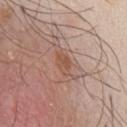The lesion-visualizer software estimated a shape eccentricity near 0.85 and a symmetry-axis asymmetry near 0.3. It also reported border irregularity of about 3 on a 0–10 scale, internal color variation of about 2 on a 0–10 scale, and peripheral color asymmetry of about 0.5.
The subject is a male aged approximately 45.
Approximately 3.5 mm at its widest.
A region of skin cropped from a whole-body photographic capture, roughly 15 mm wide.
The lesion is on the chest.